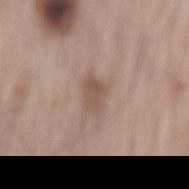The lesion was tiled from a total-body skin photograph and was not biopsied. The subject is a male aged 63–67. A 15 mm crop from a total-body photograph taken for skin-cancer surveillance. On the back. The recorded lesion diameter is about 3 mm. The tile uses white-light illumination. Automated tile analysis of the lesion measured an area of roughly 5 mm² and a shape eccentricity near 0.75. It also reported a mean CIELAB color near L≈53 a*≈16 b*≈25, a lesion–skin lightness drop of about 9, and a lesion-to-skin contrast of about 6.5 (normalized; higher = more distinct). And it measured a detector confidence of about 100 out of 100 that the crop contains a lesion.